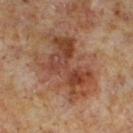Assessment:
Recorded during total-body skin imaging; not selected for excision or biopsy.